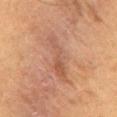This lesion was catalogued during total-body skin photography and was not selected for biopsy. The subject is a male aged 53–57. Located on the abdomen. A close-up tile cropped from a whole-body skin photograph, about 15 mm across. The tile uses cross-polarized illumination.